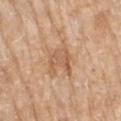{
  "biopsy_status": "not biopsied; imaged during a skin examination",
  "lesion_size": {
    "long_diameter_mm_approx": 3.5
  },
  "site": "right upper arm",
  "patient": {
    "sex": "female",
    "age_approx": 75
  },
  "image": {
    "source": "total-body photography crop",
    "field_of_view_mm": 15
  },
  "lighting": "white-light"
}